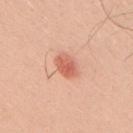notes: imaged on a skin check; not biopsied | anatomic site: the upper back | imaging modality: total-body-photography crop, ~15 mm field of view | lighting: white-light | image-analysis metrics: a footprint of about 5 mm², a shape eccentricity near 0.7, and a shape-asymmetry score of about 0.2 (0 = symmetric); a mean CIELAB color near L≈62 a*≈30 b*≈33, a lesion–skin lightness drop of about 12, and a normalized border contrast of about 7.5; an automated nevus-likeness rating near 95 out of 100 and lesion-presence confidence of about 100/100 | patient: male, aged 28 to 32 | lesion diameter: ≈3 mm.